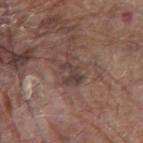follow-up = catalogued during a skin exam; not biopsied
subject = male, aged 78 to 82
lesion diameter = ≈4 mm
location = the chest
image source = total-body-photography crop, ~15 mm field of view
automated metrics = a within-lesion color-variation index near 2.5/10; lesion-presence confidence of about 90/100
tile lighting = white-light illumination